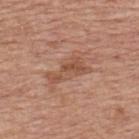Captured during whole-body skin photography for melanoma surveillance; the lesion was not biopsied. The subject is a male about 80 years old. A close-up tile cropped from a whole-body skin photograph, about 15 mm across. From the back. Imaged with white-light lighting. Longest diameter approximately 5 mm. Automated tile analysis of the lesion measured an average lesion color of about L≈51 a*≈22 b*≈31 (CIELAB), a lesion–skin lightness drop of about 9, and a normalized lesion–skin contrast near 6.5. And it measured a border-irregularity rating of about 8.5/10, a color-variation rating of about 2/10, and a peripheral color-asymmetry measure near 0.5.